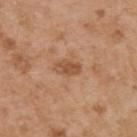The lesion was photographed on a routine skin check and not biopsied; there is no pathology result.
On the upper back.
The tile uses white-light illumination.
An algorithmic analysis of the crop reported an area of roughly 4.5 mm² and an outline eccentricity of about 0.85 (0 = round, 1 = elongated). It also reported a border-irregularity rating of about 2/10, a within-lesion color-variation index near 1.5/10, and peripheral color asymmetry of about 0.5.
The recorded lesion diameter is about 3.5 mm.
The patient is a male in their mid-50s.
A close-up tile cropped from a whole-body skin photograph, about 15 mm across.